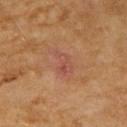This lesion was catalogued during total-body skin photography and was not selected for biopsy.
Located on the arm.
The recorded lesion diameter is about 3.5 mm.
This image is a 15 mm lesion crop taken from a total-body photograph.
The subject is a female aged 58 to 62.
Automated image analysis of the tile measured border irregularity of about 5 on a 0–10 scale, internal color variation of about 4.5 on a 0–10 scale, and a peripheral color-asymmetry measure near 1.5.